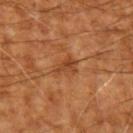- site — the left upper arm
- automated metrics — a lesion area of about 4 mm², a shape eccentricity near 0.75, and a symmetry-axis asymmetry near 0.45
- lighting — cross-polarized
- lesion diameter — ~3 mm (longest diameter)
- subject — aged 63 to 67
- image — total-body-photography crop, ~15 mm field of view